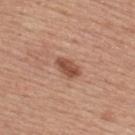workup: no biopsy performed (imaged during a skin exam); anatomic site: the upper back; automated metrics: an outline eccentricity of about 0.8 (0 = round, 1 = elongated) and a shape-asymmetry score of about 0.25 (0 = symmetric); image source: total-body-photography crop, ~15 mm field of view; lighting: white-light; patient: female, aged 63–67; lesion diameter: about 3 mm.